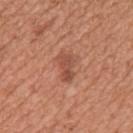Cropped from a whole-body photographic skin survey; the tile spans about 15 mm. This is a white-light tile. Longest diameter approximately 3.5 mm. Automated tile analysis of the lesion measured roughly 9 lightness units darker than nearby skin and a lesion-to-skin contrast of about 6.5 (normalized; higher = more distinct). The software also gave a border-irregularity rating of about 3.5/10 and peripheral color asymmetry of about 1. The patient is a female about 30 years old. Located on the arm.